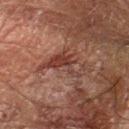| feature | finding |
|---|---|
| biopsy status | no biopsy performed (imaged during a skin exam) |
| imaging modality | total-body-photography crop, ~15 mm field of view |
| subject | male, roughly 65 years of age |
| location | the left forearm |